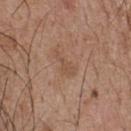workup: imaged on a skin check; not biopsied
body site: the front of the torso
patient: male, in their mid-50s
TBP lesion metrics: a shape-asymmetry score of about 0.5 (0 = symmetric); a nevus-likeness score of about 0/100 and lesion-presence confidence of about 100/100
image: ~15 mm crop, total-body skin-cancer survey
lesion diameter: about 4 mm
lighting: white-light